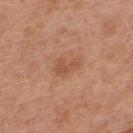Q: Was this lesion biopsied?
A: total-body-photography surveillance lesion; no biopsy
Q: Automated lesion metrics?
A: roughly 8 lightness units darker than nearby skin; a border-irregularity index near 5/10 and a within-lesion color-variation index near 1/10
Q: Lesion size?
A: about 3 mm
Q: Lesion location?
A: the upper back
Q: What kind of image is this?
A: ~15 mm crop, total-body skin-cancer survey
Q: Illumination type?
A: white-light
Q: Patient demographics?
A: male, about 30 years old